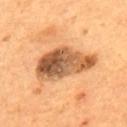Captured under cross-polarized illumination. From the back. Automated tile analysis of the lesion measured a lesion–skin lightness drop of about 17 and a normalized border contrast of about 11.5. The software also gave a border-irregularity index near 3.5/10, a within-lesion color-variation index near 10/10, and a peripheral color-asymmetry measure near 3.5. A 15 mm close-up tile from a total-body photography series done for melanoma screening. A male subject aged 53–57.